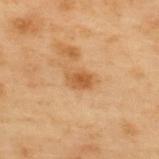Notes:
- biopsy status · catalogued during a skin exam; not biopsied
- automated metrics · an area of roughly 5 mm² and a shape-asymmetry score of about 0.15 (0 = symmetric); an average lesion color of about L≈46 a*≈19 b*≈35 (CIELAB), a lesion–skin lightness drop of about 8, and a lesion-to-skin contrast of about 7 (normalized; higher = more distinct); border irregularity of about 1.5 on a 0–10 scale, internal color variation of about 2.5 on a 0–10 scale, and a peripheral color-asymmetry measure near 1
- image · ~15 mm tile from a whole-body skin photo
- tile lighting · cross-polarized
- location · the upper back
- patient · male, aged around 55
- lesion diameter · ~3 mm (longest diameter)